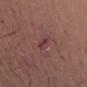Captured during whole-body skin photography for melanoma surveillance; the lesion was not biopsied. The patient is a male in their mid-40s. A roughly 15 mm field-of-view crop from a total-body skin photograph. The lesion's longest dimension is about 2.5 mm. The lesion is on the right forearm. The tile uses white-light illumination.